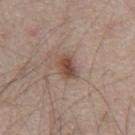Impression: Imaged during a routine full-body skin examination; the lesion was not biopsied and no histopathology is available. Acquisition and patient details: An algorithmic analysis of the crop reported a footprint of about 5.5 mm² and an eccentricity of roughly 0.65. And it measured a lesion color around L≈47 a*≈18 b*≈25 in CIELAB, roughly 11 lightness units darker than nearby skin, and a normalized border contrast of about 8.5. It also reported border irregularity of about 1.5 on a 0–10 scale, a color-variation rating of about 4.5/10, and a peripheral color-asymmetry measure near 1.5. On the abdomen. A 15 mm crop from a total-body photograph taken for skin-cancer surveillance. Imaged with white-light lighting. A male subject, about 65 years old.